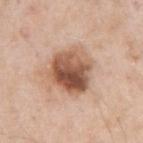Assessment: Imaged during a routine full-body skin examination; the lesion was not biopsied and no histopathology is available. Context: Automated tile analysis of the lesion measured a mean CIELAB color near L≈55 a*≈21 b*≈30. The software also gave a border-irregularity index near 2/10, a within-lesion color-variation index near 9.5/10, and a peripheral color-asymmetry measure near 4.5. A 15 mm close-up tile from a total-body photography series done for melanoma screening. From the left upper arm. A male patient, aged 53 to 57. This is a white-light tile. Longest diameter approximately 5 mm.